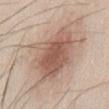Clinical impression:
The lesion was tiled from a total-body skin photograph and was not biopsied.
Background:
The lesion is on the front of the torso. This is a white-light tile. A lesion tile, about 15 mm wide, cut from a 3D total-body photograph. An algorithmic analysis of the crop reported an area of roughly 32 mm², an eccentricity of roughly 0.75, and two-axis asymmetry of about 0.25. The analysis additionally found border irregularity of about 3 on a 0–10 scale, a color-variation rating of about 5.5/10, and a peripheral color-asymmetry measure near 1.5. A male subject about 45 years old.